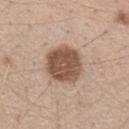Findings:
- notes: imaged on a skin check; not biopsied
- subject: female, in their 40s
- image: 15 mm crop, total-body photography
- lighting: white-light
- anatomic site: the left forearm
- TBP lesion metrics: a footprint of about 16 mm² and a shape-asymmetry score of about 0.1 (0 = symmetric); a border-irregularity rating of about 1/10, a color-variation rating of about 3/10, and a peripheral color-asymmetry measure near 1; a nevus-likeness score of about 25/100